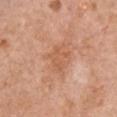Case summary:
– location · the front of the torso
– patient · female, roughly 60 years of age
– image · total-body-photography crop, ~15 mm field of view
– TBP lesion metrics · a shape eccentricity near 0.75 and a shape-asymmetry score of about 0.35 (0 = symmetric); an average lesion color of about L≈58 a*≈24 b*≈35 (CIELAB), a lesion–skin lightness drop of about 7, and a normalized border contrast of about 5.5
– lesion size · about 3 mm
– lighting · white-light